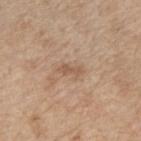| feature | finding |
|---|---|
| notes | total-body-photography surveillance lesion; no biopsy |
| location | the right upper arm |
| lesion size | about 3 mm |
| automated metrics | an area of roughly 3 mm², an outline eccentricity of about 0.9 (0 = round, 1 = elongated), and two-axis asymmetry of about 0.35; a lesion color around L≈56 a*≈18 b*≈31 in CIELAB and about 8 CIELAB-L* units darker than the surrounding skin; border irregularity of about 4 on a 0–10 scale, a within-lesion color-variation index near 0/10, and a peripheral color-asymmetry measure near 0; a nevus-likeness score of about 0/100 and lesion-presence confidence of about 100/100 |
| tile lighting | white-light |
| subject | male, aged 68–72 |
| imaging modality | 15 mm crop, total-body photography |